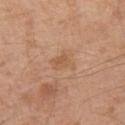The lesion was photographed on a routine skin check and not biopsied; there is no pathology result. This is a white-light tile. The subject is a male in their mid- to late 60s. A 15 mm crop from a total-body photograph taken for skin-cancer surveillance. The lesion is located on the left upper arm. Automated tile analysis of the lesion measured an eccentricity of roughly 0.65 and a symmetry-axis asymmetry near 0.4. And it measured a mean CIELAB color near L≈56 a*≈20 b*≈35, about 7 CIELAB-L* units darker than the surrounding skin, and a lesion-to-skin contrast of about 5.5 (normalized; higher = more distinct). The recorded lesion diameter is about 2.5 mm.